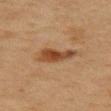Q: What are the patient's age and sex?
A: female, about 40 years old
Q: Illumination type?
A: cross-polarized
Q: What is the imaging modality?
A: ~15 mm tile from a whole-body skin photo
Q: Lesion size?
A: ~4 mm (longest diameter)
Q: What is the anatomic site?
A: the left upper arm